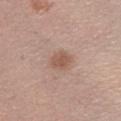Case summary:
• notes · no biopsy performed (imaged during a skin exam)
• illumination · white-light illumination
• automated metrics · a border-irregularity index near 2/10, internal color variation of about 1.5 on a 0–10 scale, and a peripheral color-asymmetry measure near 0.5; an automated nevus-likeness rating near 75 out of 100 and a lesion-detection confidence of about 100/100
• lesion diameter · ≈2.5 mm
• acquisition · ~15 mm tile from a whole-body skin photo
• body site · the left lower leg
• patient · male, aged 43–47